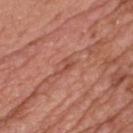| field | value |
|---|---|
| lighting | white-light illumination |
| TBP lesion metrics | a mean CIELAB color near L≈50 a*≈27 b*≈30, a lesion–skin lightness drop of about 7, and a normalized lesion–skin contrast near 5.5; border irregularity of about 5 on a 0–10 scale, a within-lesion color-variation index near 2.5/10, and radial color variation of about 0.5; a nevus-likeness score of about 0/100 and a detector confidence of about 85 out of 100 that the crop contains a lesion |
| lesion diameter | ~2.5 mm (longest diameter) |
| patient | male, aged 48 to 52 |
| image source | ~15 mm tile from a whole-body skin photo |
| body site | the chest |